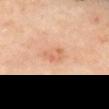Captured during whole-body skin photography for melanoma surveillance; the lesion was not biopsied. Captured under cross-polarized illumination. A female subject, aged 58–62. This image is a 15 mm lesion crop taken from a total-body photograph. From the mid back.